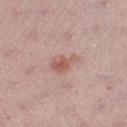Recorded during total-body skin imaging; not selected for excision or biopsy.
On the right thigh.
A female subject in their 30s.
The lesion's longest dimension is about 3.5 mm.
A roughly 15 mm field-of-view crop from a total-body skin photograph.